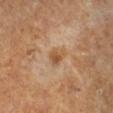<lesion>
<biopsy_status>not biopsied; imaged during a skin examination</biopsy_status>
<site>right lower leg</site>
<patient>
  <sex>female</sex>
  <age_approx>40</age_approx>
</patient>
<lesion_size>
  <long_diameter_mm_approx>2.5</long_diameter_mm_approx>
</lesion_size>
<lighting>cross-polarized</lighting>
<automated_metrics>
  <area_mm2_approx>4.0</area_mm2_approx>
  <eccentricity>0.6</eccentricity>
  <shape_asymmetry>0.25</shape_asymmetry>
  <cielab_L>53</cielab_L>
  <cielab_a>19</cielab_a>
  <cielab_b>36</cielab_b>
  <vs_skin_darker_L>9.0</vs_skin_darker_L>
  <vs_skin_contrast_norm>7.0</vs_skin_contrast_norm>
  <border_irregularity_0_10>2.0</border_irregularity_0_10>
  <color_variation_0_10>4.0</color_variation_0_10>
  <nevus_likeness_0_100>5</nevus_likeness_0_100>
  <lesion_detection_confidence_0_100>100</lesion_detection_confidence_0_100>
</automated_metrics>
<image>
  <source>total-body photography crop</source>
  <field_of_view_mm>15</field_of_view_mm>
</image>
</lesion>